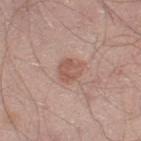workup = imaged on a skin check; not biopsied | lighting = white-light | patient = male, roughly 50 years of age | anatomic site = the left thigh | image = ~15 mm crop, total-body skin-cancer survey | automated lesion analysis = a border-irregularity rating of about 2/10, a color-variation rating of about 3/10, and peripheral color asymmetry of about 1.